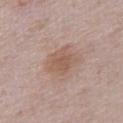The lesion was tiled from a total-body skin photograph and was not biopsied. About 3.5 mm across. A roughly 15 mm field-of-view crop from a total-body skin photograph. A male patient, approximately 40 years of age. Captured under white-light illumination. On the front of the torso. An algorithmic analysis of the crop reported an eccentricity of roughly 0.35 and a shape-asymmetry score of about 0.2 (0 = symmetric). It also reported internal color variation of about 2.5 on a 0–10 scale. And it measured a classifier nevus-likeness of about 15/100.